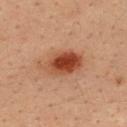workup = imaged on a skin check; not biopsied | image = ~15 mm tile from a whole-body skin photo | illumination = cross-polarized illumination | body site = the upper back | diameter = ~5.5 mm (longest diameter) | patient = male, aged 33–37 | TBP lesion metrics = a lesion area of about 13 mm² and an eccentricity of roughly 0.8; border irregularity of about 2 on a 0–10 scale, a within-lesion color-variation index near 8/10, and peripheral color asymmetry of about 2.5.